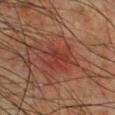Q: Was a biopsy performed?
A: imaged on a skin check; not biopsied
Q: Where on the body is the lesion?
A: the chest
Q: Patient demographics?
A: male, aged around 50
Q: What did automated image analysis measure?
A: a footprint of about 12 mm², a shape eccentricity near 0.7, and two-axis asymmetry of about 0.25; a classifier nevus-likeness of about 0/100 and a lesion-detection confidence of about 100/100
Q: What is the imaging modality?
A: ~15 mm crop, total-body skin-cancer survey
Q: What lighting was used for the tile?
A: cross-polarized illumination
Q: What is the lesion's diameter?
A: about 5 mm